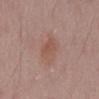{"biopsy_status": "not biopsied; imaged during a skin examination", "image": {"source": "total-body photography crop", "field_of_view_mm": 15}, "site": "mid back", "patient": {"sex": "male", "age_approx": 55}, "lesion_size": {"long_diameter_mm_approx": 3.0}}